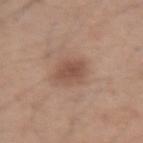Impression: The lesion was photographed on a routine skin check and not biopsied; there is no pathology result. Acquisition and patient details: Imaged with white-light lighting. The lesion is on the left upper arm. The lesion-visualizer software estimated a footprint of about 7 mm² and a shape-asymmetry score of about 0.2 (0 = symmetric). The software also gave a lesion color around L≈51 a*≈19 b*≈27 in CIELAB and about 9 CIELAB-L* units darker than the surrounding skin. The analysis additionally found lesion-presence confidence of about 100/100. This image is a 15 mm lesion crop taken from a total-body photograph. A male patient, in their mid- to late 40s.